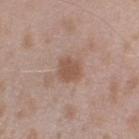Background: Approximately 2.5 mm at its widest. A lesion tile, about 15 mm wide, cut from a 3D total-body photograph. The patient is a male aged around 45. Located on the left upper arm.